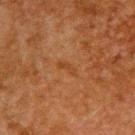Case summary:
- anatomic site · the upper back
- tile lighting · cross-polarized
- lesion size · about 2.5 mm
- image · ~15 mm tile from a whole-body skin photo
- subject · male, about 60 years old